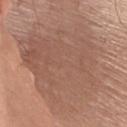workup=catalogued during a skin exam; not biopsied
lesion size=≈12 mm
subject=male, aged approximately 75
imaging modality=~15 mm crop, total-body skin-cancer survey
lighting=white-light
body site=the chest
TBP lesion metrics=peripheral color asymmetry of about 1; lesion-presence confidence of about 90/100